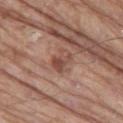<case>
  <image>
    <source>total-body photography crop</source>
    <field_of_view_mm>15</field_of_view_mm>
  </image>
  <lighting>white-light</lighting>
  <lesion_size>
    <long_diameter_mm_approx>3.0</long_diameter_mm_approx>
  </lesion_size>
  <patient>
    <sex>male</sex>
    <age_approx>80</age_approx>
  </patient>
  <site>left thigh</site>
</case>